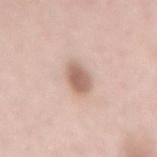lesion size: ~4 mm (longest diameter) | automated lesion analysis: a footprint of about 6.5 mm², an eccentricity of roughly 0.85, and a shape-asymmetry score of about 0.15 (0 = symmetric); an average lesion color of about L≈62 a*≈18 b*≈26 (CIELAB), about 13 CIELAB-L* units darker than the surrounding skin, and a normalized lesion–skin contrast near 8; a within-lesion color-variation index near 2.5/10 and peripheral color asymmetry of about 0.5 | acquisition: ~15 mm crop, total-body skin-cancer survey | anatomic site: the mid back | lighting: white-light illumination | subject: female, in their 50s.